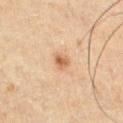patient: male, roughly 50 years of age
lighting: cross-polarized illumination
size: ≈2 mm
anatomic site: the chest
TBP lesion metrics: internal color variation of about 2 on a 0–10 scale and radial color variation of about 0.5; lesion-presence confidence of about 100/100
imaging modality: 15 mm crop, total-body photography